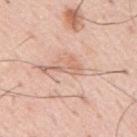Image and clinical context:
The tile uses white-light illumination. From the mid back. The recorded lesion diameter is about 3 mm. A male subject aged approximately 55. This image is a 15 mm lesion crop taken from a total-body photograph.